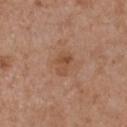notes: imaged on a skin check; not biopsied | patient: female, in their mid- to late 50s | image-analysis metrics: an area of roughly 4 mm², an outline eccentricity of about 0.8 (0 = round, 1 = elongated), and a symmetry-axis asymmetry near 0.3; a color-variation rating of about 2/10 and radial color variation of about 0.5 | tile lighting: white-light | body site: the chest | lesion diameter: about 3 mm | imaging modality: 15 mm crop, total-body photography.